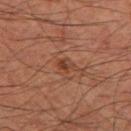Notes:
– notes: catalogued during a skin exam; not biopsied
– tile lighting: cross-polarized
– subject: male, aged 58 to 62
– body site: the leg
– imaging modality: ~15 mm crop, total-body skin-cancer survey